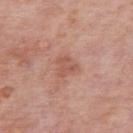{"biopsy_status": "not biopsied; imaged during a skin examination", "automated_metrics": {"nevus_likeness_0_100": 0, "lesion_detection_confidence_0_100": 100}, "site": "upper back", "patient": {"sex": "male", "age_approx": 75}, "image": {"source": "total-body photography crop", "field_of_view_mm": 15}, "lesion_size": {"long_diameter_mm_approx": 2.5}, "lighting": "white-light"}